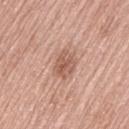Recorded during total-body skin imaging; not selected for excision or biopsy. The subject is a female aged approximately 60. The lesion is located on the leg. A 15 mm crop from a total-body photograph taken for skin-cancer surveillance. Longest diameter approximately 3.5 mm. This is a white-light tile.